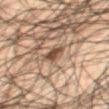Clinical impression: Recorded during total-body skin imaging; not selected for excision or biopsy. Background: On the mid back. A male patient, approximately 50 years of age. An algorithmic analysis of the crop reported an area of roughly 4 mm², an eccentricity of roughly 0.65, and a shape-asymmetry score of about 0.2 (0 = symmetric). The analysis additionally found a classifier nevus-likeness of about 85/100 and a detector confidence of about 85 out of 100 that the crop contains a lesion. Cropped from a whole-body photographic skin survey; the tile spans about 15 mm. Captured under cross-polarized illumination. About 2.5 mm across.